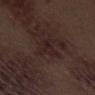follow-up: catalogued during a skin exam; not biopsied
image: ~15 mm tile from a whole-body skin photo
body site: the leg
patient: male, aged 68–72